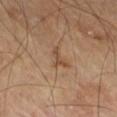biopsy_status: not biopsied; imaged during a skin examination
patient:
  sex: male
  age_approx: 70
automated_metrics:
  nevus_likeness_0_100: 0
  lesion_detection_confidence_0_100: 100
site: right thigh
image:
  source: total-body photography crop
  field_of_view_mm: 15
lesion_size:
  long_diameter_mm_approx: 3.0